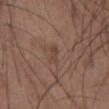Case summary:
• workup: no biopsy performed (imaged during a skin exam)
• automated lesion analysis: a lesion color around L≈43 a*≈18 b*≈27 in CIELAB, about 6 CIELAB-L* units darker than the surrounding skin, and a lesion-to-skin contrast of about 5 (normalized; higher = more distinct); a within-lesion color-variation index near 0/10 and radial color variation of about 0; a nevus-likeness score of about 0/100
• lighting: white-light
• image: ~15 mm crop, total-body skin-cancer survey
• subject: male, roughly 55 years of age
• site: the abdomen
• lesion diameter: about 2.5 mm